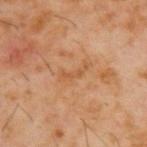<case>
<patient>
  <sex>male</sex>
  <age_approx>60</age_approx>
</patient>
<lesion_size>
  <long_diameter_mm_approx>3.5</long_diameter_mm_approx>
</lesion_size>
<lighting>cross-polarized</lighting>
<image>
  <source>total-body photography crop</source>
  <field_of_view_mm>15</field_of_view_mm>
</image>
<site>upper back</site>
</case>